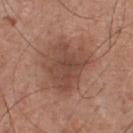<record>
<biopsy_status>not biopsied; imaged during a skin examination</biopsy_status>
<site>chest</site>
<lighting>white-light</lighting>
<image>
  <source>total-body photography crop</source>
  <field_of_view_mm>15</field_of_view_mm>
</image>
<patient>
  <sex>male</sex>
  <age_approx>55</age_approx>
</patient>
</record>